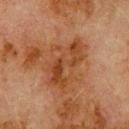Assessment: This lesion was catalogued during total-body skin photography and was not selected for biopsy. Background: Captured under cross-polarized illumination. The lesion is on the upper back. A male patient aged 78 to 82. Longest diameter approximately 6.5 mm. Cropped from a whole-body photographic skin survey; the tile spans about 15 mm.